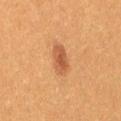The lesion was photographed on a routine skin check and not biopsied; there is no pathology result. Automated image analysis of the tile measured a footprint of about 6.5 mm² and a shape eccentricity near 0.8. It also reported a lesion color around L≈45 a*≈22 b*≈33 in CIELAB and roughly 9 lightness units darker than nearby skin. It also reported a within-lesion color-variation index near 3/10 and a peripheral color-asymmetry measure near 1. The recorded lesion diameter is about 3.5 mm. The patient is a female aged 38–42. The lesion is located on the right thigh. Cropped from a whole-body photographic skin survey; the tile spans about 15 mm. Captured under cross-polarized illumination.